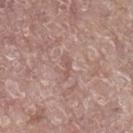Q: Was a biopsy performed?
A: no biopsy performed (imaged during a skin exam)